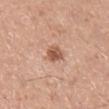The lesion was photographed on a routine skin check and not biopsied; there is no pathology result. On the right lower leg. About 2.5 mm across. The total-body-photography lesion software estimated an average lesion color of about L≈56 a*≈22 b*≈31 (CIELAB), roughly 13 lightness units darker than nearby skin, and a normalized border contrast of about 8.5. The analysis additionally found a border-irregularity rating of about 2/10 and peripheral color asymmetry of about 1. The subject is a male aged around 60. Cropped from a whole-body photographic skin survey; the tile spans about 15 mm.